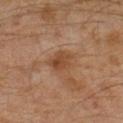{"automated_metrics": {"border_irregularity_0_10": 2.0}, "site": "left leg", "lighting": "cross-polarized", "lesion_size": {"long_diameter_mm_approx": 3.0}, "patient": {"sex": "male", "age_approx": 30}, "image": {"source": "total-body photography crop", "field_of_view_mm": 15}}